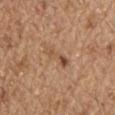No biopsy was performed on this lesion — it was imaged during a full skin examination and was not determined to be concerning.
The recorded lesion diameter is about 4 mm.
Located on the head or neck.
A roughly 15 mm field-of-view crop from a total-body skin photograph.
Automated tile analysis of the lesion measured a lesion area of about 4.5 mm², an eccentricity of roughly 0.95, and a symmetry-axis asymmetry near 0.3. It also reported a mean CIELAB color near L≈52 a*≈19 b*≈32, about 9 CIELAB-L* units darker than the surrounding skin, and a normalized border contrast of about 6.5. The analysis additionally found a classifier nevus-likeness of about 0/100 and lesion-presence confidence of about 100/100.
Imaged with white-light lighting.
A male subject aged 68–72.